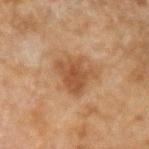Captured during whole-body skin photography for melanoma surveillance; the lesion was not biopsied. The lesion is on the left forearm. A close-up tile cropped from a whole-body skin photograph, about 15 mm across. The subject is a male aged 43–47.